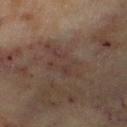{"biopsy_status": "not biopsied; imaged during a skin examination", "image": {"source": "total-body photography crop", "field_of_view_mm": 15}, "automated_metrics": {"cielab_L": 33, "cielab_a": 15, "cielab_b": 19, "border_irregularity_0_10": 5.5, "color_variation_0_10": 3.0, "peripheral_color_asymmetry": 1.0}, "patient": {"sex": "female", "age_approx": 60}, "site": "left lower leg", "lesion_size": {"long_diameter_mm_approx": 5.0}, "lighting": "cross-polarized"}